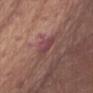follow-up = catalogued during a skin exam; not biopsied
image = ~15 mm crop, total-body skin-cancer survey
body site = the front of the torso
subject = male, aged around 70
lesion diameter = about 3.5 mm